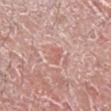Assessment: This lesion was catalogued during total-body skin photography and was not selected for biopsy. Acquisition and patient details: A lesion tile, about 15 mm wide, cut from a 3D total-body photograph. Automated tile analysis of the lesion measured a shape eccentricity near 0.85 and a shape-asymmetry score of about 0.3 (0 = symmetric). The tile uses white-light illumination. A male patient, aged 18 to 22. The recorded lesion diameter is about 2.5 mm. From the right lower leg.